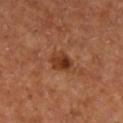follow-up = total-body-photography surveillance lesion; no biopsy
image = 15 mm crop, total-body photography
lighting = cross-polarized
image-analysis metrics = an area of roughly 6 mm², a shape eccentricity near 0.55, and a symmetry-axis asymmetry near 0.2; an average lesion color of about L≈38 a*≈25 b*≈33 (CIELAB), roughly 10 lightness units darker than nearby skin, and a normalized lesion–skin contrast near 8.5; a border-irregularity index near 2/10, internal color variation of about 6.5 on a 0–10 scale, and radial color variation of about 2.5; a classifier nevus-likeness of about 80/100 and a lesion-detection confidence of about 100/100
subject = male, aged 63 to 67
size = about 3 mm
anatomic site = the right lower leg